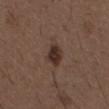This lesion was catalogued during total-body skin photography and was not selected for biopsy. A male subject in their 50s. A 15 mm close-up tile from a total-body photography series done for melanoma screening. From the chest.